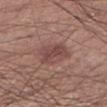| feature | finding |
|---|---|
| workup | no biopsy performed (imaged during a skin exam) |
| automated lesion analysis | an area of roughly 9 mm², an eccentricity of roughly 0.65, and two-axis asymmetry of about 0.2; a lesion–skin lightness drop of about 9 and a normalized border contrast of about 7 |
| size | about 3.5 mm |
| image | ~15 mm crop, total-body skin-cancer survey |
| location | the right lower leg |
| patient | male, aged approximately 45 |